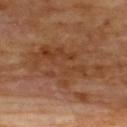Findings:
• follow-up: catalogued during a skin exam; not biopsied
• subject: male, about 70 years old
• automated lesion analysis: a footprint of about 26 mm² and two-axis asymmetry of about 0.45; an average lesion color of about L≈40 a*≈21 b*≈32 (CIELAB), a lesion–skin lightness drop of about 7, and a normalized lesion–skin contrast near 6; a color-variation rating of about 4/10; lesion-presence confidence of about 95/100
• body site: the upper back
• lesion diameter: about 9 mm
• tile lighting: cross-polarized
• image source: ~15 mm tile from a whole-body skin photo